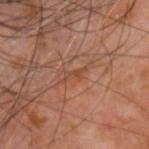<record>
<biopsy_status>not biopsied; imaged during a skin examination</biopsy_status>
<lighting>cross-polarized</lighting>
<lesion_size>
  <long_diameter_mm_approx>3.0</long_diameter_mm_approx>
</lesion_size>
<site>head or neck</site>
<image>
  <source>total-body photography crop</source>
  <field_of_view_mm>15</field_of_view_mm>
</image>
<patient>
  <sex>male</sex>
  <age_approx>60</age_approx>
</patient>
</record>